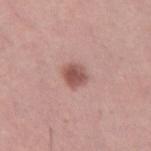Notes:
• notes — catalogued during a skin exam; not biopsied
• image-analysis metrics — an outline eccentricity of about 0.45 (0 = round, 1 = elongated) and a shape-asymmetry score of about 0.2 (0 = symmetric); border irregularity of about 2 on a 0–10 scale, internal color variation of about 3.5 on a 0–10 scale, and a peripheral color-asymmetry measure near 1; a classifier nevus-likeness of about 90/100 and a detector confidence of about 100 out of 100 that the crop contains a lesion
• diameter — ≈3 mm
• lighting — white-light
• subject — female, in their 40s
• location — the right thigh
• acquisition — 15 mm crop, total-body photography